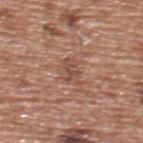Captured during whole-body skin photography for melanoma surveillance; the lesion was not biopsied.
A close-up tile cropped from a whole-body skin photograph, about 15 mm across.
Approximately 3 mm at its widest.
On the upper back.
Automated image analysis of the tile measured an average lesion color of about L≈49 a*≈22 b*≈27 (CIELAB), about 8 CIELAB-L* units darker than the surrounding skin, and a normalized border contrast of about 6. The analysis additionally found a classifier nevus-likeness of about 0/100.
A male patient, aged approximately 75.